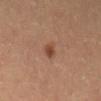Impression: The lesion was tiled from a total-body skin photograph and was not biopsied. Background: The lesion is located on the abdomen. The tile uses cross-polarized illumination. Approximately 2.5 mm at its widest. This image is a 15 mm lesion crop taken from a total-body photograph. A female patient, aged approximately 60.